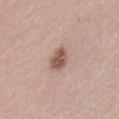Clinical impression: Imaged during a routine full-body skin examination; the lesion was not biopsied and no histopathology is available. Background: A female patient, roughly 65 years of age. Measured at roughly 3.5 mm in maximum diameter. This image is a 15 mm lesion crop taken from a total-body photograph. On the lower back. The tile uses white-light illumination.